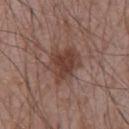| field | value |
|---|---|
| biopsy status | total-body-photography surveillance lesion; no biopsy |
| acquisition | ~15 mm crop, total-body skin-cancer survey |
| subject | male, aged around 70 |
| diameter | ≈4.5 mm |
| TBP lesion metrics | a footprint of about 11 mm², an outline eccentricity of about 0.6 (0 = round, 1 = elongated), and a shape-asymmetry score of about 0.25 (0 = symmetric); a detector confidence of about 100 out of 100 that the crop contains a lesion |
| site | the chest |